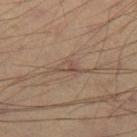Part of a total-body skin-imaging series; this lesion was reviewed on a skin check and was not flagged for biopsy. A region of skin cropped from a whole-body photographic capture, roughly 15 mm wide. The patient is a male aged around 40. On the right thigh. Captured under cross-polarized illumination.